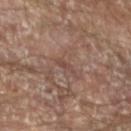  biopsy_status: not biopsied; imaged during a skin examination
  image:
    source: total-body photography crop
    field_of_view_mm: 15
  lesion_size:
    long_diameter_mm_approx: 2.5
  patient:
    sex: male
    age_approx: 65
  automated_metrics:
    cielab_L: 44
    cielab_a: 18
    cielab_b: 22
    vs_skin_darker_L: 6.0
    vs_skin_contrast_norm: 5.0
  site: arm
  lighting: cross-polarized